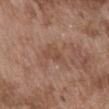Clinical impression:
Captured during whole-body skin photography for melanoma surveillance; the lesion was not biopsied.
Context:
Captured under white-light illumination. A close-up tile cropped from a whole-body skin photograph, about 15 mm across. The patient is a male aged 73 to 77. The lesion is located on the front of the torso.